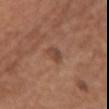Impression:
Captured during whole-body skin photography for melanoma surveillance; the lesion was not biopsied.
Clinical summary:
A female subject, about 75 years old. The tile uses white-light illumination. A region of skin cropped from a whole-body photographic capture, roughly 15 mm wide. The lesion is located on the front of the torso.